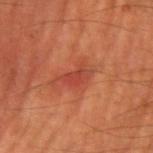biopsy status: catalogued during a skin exam; not biopsied | tile lighting: cross-polarized | body site: the right upper arm | acquisition: 15 mm crop, total-body photography | subject: male, in their mid-60s.